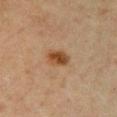The lesion was photographed on a routine skin check and not biopsied; there is no pathology result. Measured at roughly 3 mm in maximum diameter. The subject is a female about 40 years old. From the chest. A region of skin cropped from a whole-body photographic capture, roughly 15 mm wide.